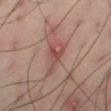Impression: Recorded during total-body skin imaging; not selected for excision or biopsy. Background: On the right thigh. Imaged with cross-polarized lighting. Cropped from a total-body skin-imaging series; the visible field is about 15 mm. A male patient, aged 38–42.